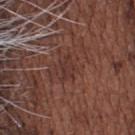Q: Was a biopsy performed?
A: catalogued during a skin exam; not biopsied
Q: What did automated image analysis measure?
A: a footprint of about 3 mm², an eccentricity of roughly 0.8, and a shape-asymmetry score of about 0.35 (0 = symmetric); a nevus-likeness score of about 0/100 and lesion-presence confidence of about 90/100
Q: How was the tile lit?
A: white-light
Q: What is the anatomic site?
A: the head or neck
Q: How large is the lesion?
A: ~2.5 mm (longest diameter)
Q: Who is the patient?
A: male, aged 73 to 77
Q: How was this image acquired?
A: 15 mm crop, total-body photography